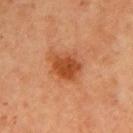Q: Was a biopsy performed?
A: catalogued during a skin exam; not biopsied
Q: What is the anatomic site?
A: the left upper arm
Q: Patient demographics?
A: female, aged approximately 70
Q: How was the tile lit?
A: cross-polarized
Q: What kind of image is this?
A: total-body-photography crop, ~15 mm field of view
Q: What is the lesion's diameter?
A: about 4 mm